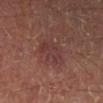The lesion was tiled from a total-body skin photograph and was not biopsied. A roughly 15 mm field-of-view crop from a total-body skin photograph. Automated tile analysis of the lesion measured a footprint of about 8.5 mm², an eccentricity of roughly 0.8, and a shape-asymmetry score of about 0.3 (0 = symmetric). And it measured a mean CIELAB color near L≈32 a*≈19 b*≈19, about 5 CIELAB-L* units darker than the surrounding skin, and a normalized lesion–skin contrast near 5.5. The software also gave border irregularity of about 4 on a 0–10 scale, internal color variation of about 3 on a 0–10 scale, and radial color variation of about 1. The software also gave a nevus-likeness score of about 5/100 and a detector confidence of about 100 out of 100 that the crop contains a lesion. Imaged with cross-polarized lighting. A male patient, approximately 50 years of age. The lesion's longest dimension is about 4.5 mm. Located on the right lower leg.